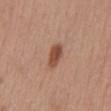{
  "site": "mid back",
  "lesion_size": {
    "long_diameter_mm_approx": 3.0
  },
  "image": {
    "source": "total-body photography crop",
    "field_of_view_mm": 15
  },
  "patient": {
    "sex": "female",
    "age_approx": 55
  }
}